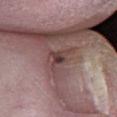{"biopsy_status": "not biopsied; imaged during a skin examination", "automated_metrics": {"cielab_L": 42, "cielab_a": 18, "cielab_b": 18, "vs_skin_darker_L": 9.0, "lesion_detection_confidence_0_100": 55}, "lighting": "white-light", "image": {"source": "total-body photography crop", "field_of_view_mm": 15}, "patient": {"sex": "male", "age_approx": 80}, "site": "leg", "lesion_size": {"long_diameter_mm_approx": 4.5}}